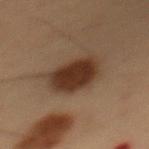This lesion was catalogued during total-body skin photography and was not selected for biopsy.
The lesion is located on the mid back.
Captured under cross-polarized illumination.
About 5.5 mm across.
A male subject, about 55 years old.
A 15 mm close-up extracted from a 3D total-body photography capture.